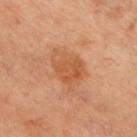The lesion was tiled from a total-body skin photograph and was not biopsied.
The subject is a female in their mid- to late 50s.
Captured under cross-polarized illumination.
A roughly 15 mm field-of-view crop from a total-body skin photograph.
From the front of the torso.